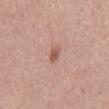workup: catalogued during a skin exam; not biopsied
image: total-body-photography crop, ~15 mm field of view
tile lighting: white-light
body site: the mid back
automated lesion analysis: an area of roughly 2.5 mm², an eccentricity of roughly 0.85, and a symmetry-axis asymmetry near 0.2; a lesion color around L≈56 a*≈23 b*≈27 in CIELAB and a lesion-to-skin contrast of about 7 (normalized; higher = more distinct); a border-irregularity index near 2/10, a within-lesion color-variation index near 1.5/10, and peripheral color asymmetry of about 0.5; a nevus-likeness score of about 35/100 and a detector confidence of about 100 out of 100 that the crop contains a lesion
patient: female, in their mid-60s
diameter: ≈2.5 mm